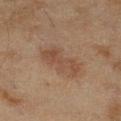– biopsy status — imaged on a skin check; not biopsied
– illumination — cross-polarized
– anatomic site — the right lower leg
– image — ~15 mm tile from a whole-body skin photo
– image-analysis metrics — a border-irregularity index near 5.5/10, internal color variation of about 3 on a 0–10 scale, and a peripheral color-asymmetry measure near 1; an automated nevus-likeness rating near 20 out of 100
– subject — female, approximately 60 years of age